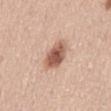Image and clinical context:
Located on the back. Automated tile analysis of the lesion measured a lesion color around L≈57 a*≈22 b*≈29 in CIELAB and about 16 CIELAB-L* units darker than the surrounding skin. The software also gave a border-irregularity index near 2/10 and radial color variation of about 1.5. It also reported a lesion-detection confidence of about 100/100. About 4.5 mm across. A male subject aged 63–67. The tile uses white-light illumination. A 15 mm close-up tile from a total-body photography series done for melanoma screening.